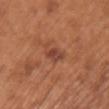workup=total-body-photography surveillance lesion; no biopsy | imaging modality=~15 mm tile from a whole-body skin photo | automated lesion analysis=a lesion area of about 3.5 mm² and an eccentricity of roughly 0.8; border irregularity of about 3 on a 0–10 scale, a color-variation rating of about 2.5/10, and radial color variation of about 1; a detector confidence of about 100 out of 100 that the crop contains a lesion | diameter=about 2.5 mm | illumination=white-light | location=the chest | subject=female, aged 63 to 67.